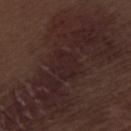Assessment:
Imaged during a routine full-body skin examination; the lesion was not biopsied and no histopathology is available.
Acquisition and patient details:
The patient is a male approximately 70 years of age. Automated image analysis of the tile measured a footprint of about 8.5 mm² and a symmetry-axis asymmetry near 0.2. A lesion tile, about 15 mm wide, cut from a 3D total-body photograph. The lesion is on the leg. Longest diameter approximately 3.5 mm.